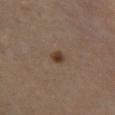Case summary:
– workup · no biopsy performed (imaged during a skin exam)
– imaging modality · ~15 mm crop, total-body skin-cancer survey
– automated metrics · an area of roughly 2.5 mm² and a shape eccentricity near 0.6; border irregularity of about 2.5 on a 0–10 scale, internal color variation of about 3.5 on a 0–10 scale, and peripheral color asymmetry of about 1.5; a nevus-likeness score of about 100/100 and a detector confidence of about 100 out of 100 that the crop contains a lesion
– subject · male, about 50 years old
– site · the left lower leg
– diameter · ≈2 mm